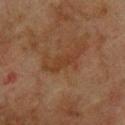<tbp_lesion>
  <biopsy_status>not biopsied; imaged during a skin examination</biopsy_status>
  <image>
    <source>total-body photography crop</source>
    <field_of_view_mm>15</field_of_view_mm>
  </image>
  <site>upper back</site>
  <patient>
    <sex>male</sex>
    <age_approx>75</age_approx>
  </patient>
  <lighting>cross-polarized</lighting>
  <lesion_size>
    <long_diameter_mm_approx>3.5</long_diameter_mm_approx>
  </lesion_size>
</tbp_lesion>